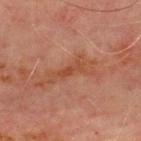This lesion was catalogued during total-body skin photography and was not selected for biopsy. The recorded lesion diameter is about 3 mm. From the chest. The tile uses cross-polarized illumination. The patient is a male about 70 years old. The total-body-photography lesion software estimated a footprint of about 2.5 mm², a shape eccentricity near 0.95, and two-axis asymmetry of about 0.35. The software also gave an average lesion color of about L≈38 a*≈23 b*≈29 (CIELAB) and a lesion–skin lightness drop of about 6. It also reported a border-irregularity rating of about 4.5/10, a color-variation rating of about 0/10, and radial color variation of about 0. And it measured a nevus-likeness score of about 0/100 and a detector confidence of about 90 out of 100 that the crop contains a lesion. A region of skin cropped from a whole-body photographic capture, roughly 15 mm wide.